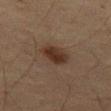follow-up = total-body-photography surveillance lesion; no biopsy | TBP lesion metrics = an eccentricity of roughly 0.8 and two-axis asymmetry of about 0.15; a lesion color around L≈28 a*≈15 b*≈23 in CIELAB and about 9 CIELAB-L* units darker than the surrounding skin | size = ~3.5 mm (longest diameter) | location = the left thigh | subject = female, approximately 55 years of age | tile lighting = cross-polarized illumination | acquisition = ~15 mm crop, total-body skin-cancer survey.